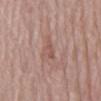notes: no biopsy performed (imaged during a skin exam) | size: about 3 mm | acquisition: ~15 mm tile from a whole-body skin photo | lighting: white-light illumination | automated lesion analysis: a lesion color around L≈53 a*≈21 b*≈24 in CIELAB | patient: female, aged around 65 | site: the back.